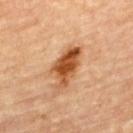follow-up: catalogued during a skin exam; not biopsied
lesion size: ≈5.5 mm
automated metrics: a lesion area of about 11 mm², a shape eccentricity near 0.85, and a shape-asymmetry score of about 0.3 (0 = symmetric); an average lesion color of about L≈51 a*≈26 b*≈40 (CIELAB) and a normalized lesion–skin contrast near 11
image: ~15 mm tile from a whole-body skin photo
patient: male, aged 83 or over
body site: the upper back
illumination: cross-polarized illumination